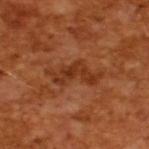Notes:
• follow-up — no biopsy performed (imaged during a skin exam)
• tile lighting — cross-polarized illumination
• size — about 6 mm
• image-analysis metrics — a lesion–skin lightness drop of about 8 and a normalized lesion–skin contrast near 7
• acquisition — 15 mm crop, total-body photography
• subject — male, in their mid-60s